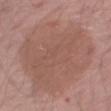Q: Was a biopsy performed?
A: imaged on a skin check; not biopsied
Q: What is the anatomic site?
A: the right thigh
Q: What did automated image analysis measure?
A: border irregularity of about 2.5 on a 0–10 scale, a within-lesion color-variation index near 3/10, and radial color variation of about 1
Q: How was this image acquired?
A: 15 mm crop, total-body photography
Q: Illumination type?
A: white-light
Q: Lesion size?
A: ~11.5 mm (longest diameter)
Q: What are the patient's age and sex?
A: male, aged around 65